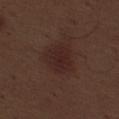Recorded during total-body skin imaging; not selected for excision or biopsy.
Located on the left thigh.
Cropped from a whole-body photographic skin survey; the tile spans about 15 mm.
A male subject, roughly 70 years of age.